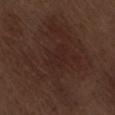Q: Is there a histopathology result?
A: imaged on a skin check; not biopsied
Q: Patient demographics?
A: male, roughly 70 years of age
Q: What is the imaging modality?
A: 15 mm crop, total-body photography
Q: Lesion size?
A: ~11.5 mm (longest diameter)
Q: Lesion location?
A: the lower back
Q: What lighting was used for the tile?
A: white-light